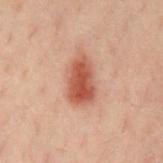{"biopsy_status": "not biopsied; imaged during a skin examination", "patient": {"sex": "male", "age_approx": 40}, "site": "chest", "image": {"source": "total-body photography crop", "field_of_view_mm": 15}, "lesion_size": {"long_diameter_mm_approx": 5.0}}